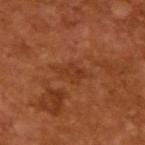No biopsy was performed on this lesion — it was imaged during a full skin examination and was not determined to be concerning. A roughly 15 mm field-of-view crop from a total-body skin photograph. A male patient, aged 63 to 67. The total-body-photography lesion software estimated a footprint of about 7 mm² and an eccentricity of roughly 0.85. Longest diameter approximately 4.5 mm. Imaged with cross-polarized lighting.